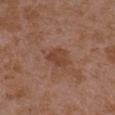follow-up: imaged on a skin check; not biopsied
image: total-body-photography crop, ~15 mm field of view
tile lighting: white-light illumination
anatomic site: the arm
subject: female, in their mid-30s
diameter: ≈3.5 mm